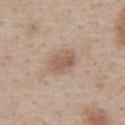Assessment: Recorded during total-body skin imaging; not selected for excision or biopsy. Image and clinical context: On the chest. A male patient aged 58 to 62. Longest diameter approximately 3.5 mm. Cropped from a whole-body photographic skin survey; the tile spans about 15 mm. Imaged with white-light lighting. Automated image analysis of the tile measured an area of roughly 8.5 mm², a shape eccentricity near 0.65, and a shape-asymmetry score of about 0.15 (0 = symmetric). And it measured an average lesion color of about L≈58 a*≈16 b*≈28 (CIELAB) and a lesion–skin lightness drop of about 9. The software also gave border irregularity of about 1 on a 0–10 scale and a within-lesion color-variation index near 3.5/10.